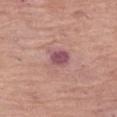workup: total-body-photography surveillance lesion; no biopsy | automated metrics: a border-irregularity index near 2.5/10, a color-variation rating of about 3/10, and radial color variation of about 1 | imaging modality: total-body-photography crop, ~15 mm field of view | size: ≈3 mm | location: the left thigh | illumination: white-light illumination | patient: female, roughly 65 years of age.